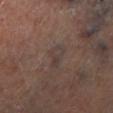<case>
  <biopsy_status>not biopsied; imaged during a skin examination</biopsy_status>
  <lighting>cross-polarized</lighting>
  <site>left lower leg</site>
  <automated_metrics>
    <lesion_detection_confidence_0_100>80</lesion_detection_confidence_0_100>
  </automated_metrics>
  <image>
    <source>total-body photography crop</source>
    <field_of_view_mm>15</field_of_view_mm>
  </image>
</case>